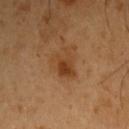This lesion was catalogued during total-body skin photography and was not selected for biopsy. Imaged with cross-polarized lighting. On the right upper arm. A male patient, about 50 years old. A 15 mm close-up tile from a total-body photography series done for melanoma screening.